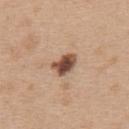This lesion was catalogued during total-body skin photography and was not selected for biopsy.
On the upper back.
The lesion-visualizer software estimated a mean CIELAB color near L≈49 a*≈20 b*≈29, a lesion–skin lightness drop of about 18, and a normalized border contrast of about 12.5.
The patient is a female in their mid-40s.
Cropped from a total-body skin-imaging series; the visible field is about 15 mm.